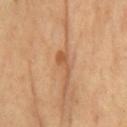Assessment: No biopsy was performed on this lesion — it was imaged during a full skin examination and was not determined to be concerning. Acquisition and patient details: The lesion's longest dimension is about 3.5 mm. The subject is a male roughly 70 years of age. Located on the chest. A 15 mm crop from a total-body photograph taken for skin-cancer surveillance.